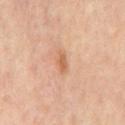This lesion was catalogued during total-body skin photography and was not selected for biopsy.
The lesion is located on the chest.
A 15 mm crop from a total-body photograph taken for skin-cancer surveillance.
Captured under cross-polarized illumination.
A male subject, approximately 65 years of age.
The lesion-visualizer software estimated a border-irregularity rating of about 2.5/10, a within-lesion color-variation index near 1.5/10, and radial color variation of about 0.5. The software also gave an automated nevus-likeness rating near 35 out of 100 and a lesion-detection confidence of about 100/100.
Approximately 2.5 mm at its widest.